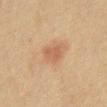From the mid back. The subject is a female approximately 70 years of age. A close-up tile cropped from a whole-body skin photograph, about 15 mm across.